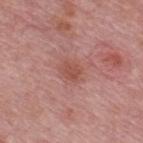Q: Is there a histopathology result?
A: imaged on a skin check; not biopsied